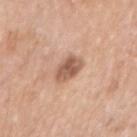Imaged during a routine full-body skin examination; the lesion was not biopsied and no histopathology is available. The patient is a male roughly 75 years of age. The lesion is located on the right upper arm. A roughly 15 mm field-of-view crop from a total-body skin photograph. The total-body-photography lesion software estimated an eccentricity of roughly 0.65 and two-axis asymmetry of about 0.2. It also reported an average lesion color of about L≈57 a*≈21 b*≈30 (CIELAB), a lesion–skin lightness drop of about 13, and a normalized border contrast of about 8.5. The software also gave a border-irregularity index near 1.5/10, a within-lesion color-variation index near 3.5/10, and radial color variation of about 1.5. And it measured lesion-presence confidence of about 100/100. Approximately 3 mm at its widest.